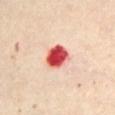Q: How was this image acquired?
A: 15 mm crop, total-body photography
Q: Where on the body is the lesion?
A: the chest
Q: What are the patient's age and sex?
A: female, aged approximately 60